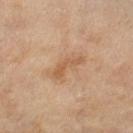The lesion was tiled from a total-body skin photograph and was not biopsied.
Located on the right lower leg.
Cropped from a total-body skin-imaging series; the visible field is about 15 mm.
An algorithmic analysis of the crop reported an automated nevus-likeness rating near 0 out of 100 and a detector confidence of about 100 out of 100 that the crop contains a lesion.
Imaged with cross-polarized lighting.
The recorded lesion diameter is about 4 mm.
A female patient roughly 60 years of age.